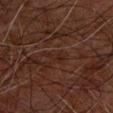Case summary:
– biopsy status · total-body-photography surveillance lesion; no biopsy
– imaging modality · 15 mm crop, total-body photography
– automated lesion analysis · an area of roughly 3.5 mm², an outline eccentricity of about 0.85 (0 = round, 1 = elongated), and a symmetry-axis asymmetry near 0.55; a border-irregularity rating of about 6/10, a within-lesion color-variation index near 1.5/10, and a peripheral color-asymmetry measure near 0.5
– patient · male, aged 78–82
– anatomic site · the right forearm
– lesion size · about 3 mm